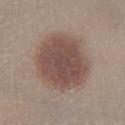Q: Is there a histopathology result?
A: no biopsy performed (imaged during a skin exam)
Q: What is the anatomic site?
A: the right lower leg
Q: Patient demographics?
A: female, aged around 45
Q: What kind of image is this?
A: ~15 mm tile from a whole-body skin photo
Q: Automated lesion metrics?
A: border irregularity of about 1.5 on a 0–10 scale, internal color variation of about 3.5 on a 0–10 scale, and a peripheral color-asymmetry measure near 1
Q: What lighting was used for the tile?
A: white-light illumination